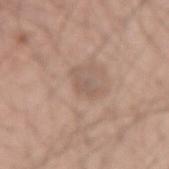This lesion was catalogued during total-body skin photography and was not selected for biopsy.
The total-body-photography lesion software estimated a border-irregularity rating of about 5.5/10 and a peripheral color-asymmetry measure near 0.5. The software also gave a nevus-likeness score of about 0/100 and a detector confidence of about 100 out of 100 that the crop contains a lesion.
Captured under white-light illumination.
A male patient, aged 53–57.
About 3.5 mm across.
A region of skin cropped from a whole-body photographic capture, roughly 15 mm wide.
The lesion is on the right forearm.